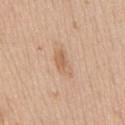Part of a total-body skin-imaging series; this lesion was reviewed on a skin check and was not flagged for biopsy. The lesion's longest dimension is about 4 mm. Cropped from a total-body skin-imaging series; the visible field is about 15 mm. A female subject, aged 73 to 77. On the front of the torso. Automated image analysis of the tile measured an area of roughly 5.5 mm², a shape eccentricity near 0.9, and a symmetry-axis asymmetry near 0.3. And it measured a lesion–skin lightness drop of about 8 and a lesion-to-skin contrast of about 5.5 (normalized; higher = more distinct). The software also gave a classifier nevus-likeness of about 15/100 and a detector confidence of about 100 out of 100 that the crop contains a lesion.